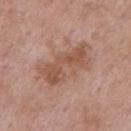This lesion was catalogued during total-body skin photography and was not selected for biopsy. Approximately 7 mm at its widest. From the right upper arm. A close-up tile cropped from a whole-body skin photograph, about 15 mm across. An algorithmic analysis of the crop reported a lesion color around L≈53 a*≈21 b*≈29 in CIELAB and a lesion-to-skin contrast of about 7 (normalized; higher = more distinct). It also reported a color-variation rating of about 4.5/10 and radial color variation of about 1.5. The patient is a male aged 53–57. This is a white-light tile.